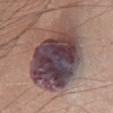Q: Is there a histopathology result?
A: catalogued during a skin exam; not biopsied
Q: How large is the lesion?
A: ~10.5 mm (longest diameter)
Q: Illumination type?
A: white-light
Q: Who is the patient?
A: male, aged 73 to 77
Q: What did automated image analysis measure?
A: a mean CIELAB color near L≈42 a*≈17 b*≈14, a lesion–skin lightness drop of about 17, and a normalized lesion–skin contrast near 15; a border-irregularity rating of about 2/10 and peripheral color asymmetry of about 3.5; a nevus-likeness score of about 0/100 and a lesion-detection confidence of about 95/100
Q: Where on the body is the lesion?
A: the front of the torso
Q: What is the imaging modality?
A: ~15 mm tile from a whole-body skin photo